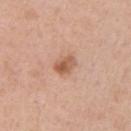{"biopsy_status": "not biopsied; imaged during a skin examination", "image": {"source": "total-body photography crop", "field_of_view_mm": 15}, "lighting": "white-light", "site": "left upper arm", "patient": {"sex": "male", "age_approx": 60}}